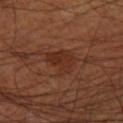This lesion was catalogued during total-body skin photography and was not selected for biopsy.
From the right thigh.
An algorithmic analysis of the crop reported an area of roughly 9 mm², an outline eccentricity of about 0.3 (0 = round, 1 = elongated), and two-axis asymmetry of about 0.4.
A male subject, in their 70s.
A 15 mm crop from a total-body photograph taken for skin-cancer surveillance.
The tile uses cross-polarized illumination.
Longest diameter approximately 3.5 mm.